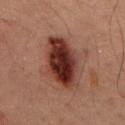Case summary:
• notes · imaged on a skin check; not biopsied
• imaging modality · ~15 mm tile from a whole-body skin photo
• illumination · cross-polarized
• patient · male, roughly 50 years of age
• anatomic site · the mid back
• size · about 6.5 mm
• automated metrics · a footprint of about 18 mm², an eccentricity of roughly 0.85, and two-axis asymmetry of about 0.15; a lesion–skin lightness drop of about 15 and a lesion-to-skin contrast of about 15 (normalized; higher = more distinct); a border-irregularity rating of about 2/10, a color-variation rating of about 5.5/10, and radial color variation of about 2; a classifier nevus-likeness of about 95/100 and a detector confidence of about 100 out of 100 that the crop contains a lesion